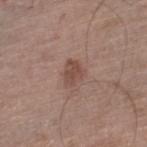Impression:
Captured during whole-body skin photography for melanoma surveillance; the lesion was not biopsied.
Clinical summary:
An algorithmic analysis of the crop reported a border-irregularity rating of about 2.5/10 and a color-variation rating of about 1.5/10. And it measured a lesion-detection confidence of about 100/100. Located on the left lower leg. A region of skin cropped from a whole-body photographic capture, roughly 15 mm wide. The lesion's longest dimension is about 3 mm. The patient is a male aged approximately 65. Imaged with white-light lighting.